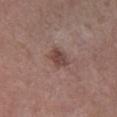Clinical impression:
Imaged during a routine full-body skin examination; the lesion was not biopsied and no histopathology is available.
Clinical summary:
A male subject, aged 53 to 57. The lesion-visualizer software estimated a footprint of about 4.5 mm² and a shape eccentricity near 0.65. And it measured a mean CIELAB color near L≈43 a*≈19 b*≈21, a lesion–skin lightness drop of about 10, and a normalized lesion–skin contrast near 8. And it measured a border-irregularity rating of about 2.5/10 and a within-lesion color-variation index near 3/10. And it measured a nevus-likeness score of about 30/100. A close-up tile cropped from a whole-body skin photograph, about 15 mm across. The lesion is located on the right lower leg. The lesion's longest dimension is about 2.5 mm.